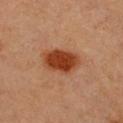<tbp_lesion>
<biopsy_status>not biopsied; imaged during a skin examination</biopsy_status>
<site>chest</site>
<patient>
  <sex>female</sex>
  <age_approx>45</age_approx>
</patient>
<image>
  <source>total-body photography crop</source>
  <field_of_view_mm>15</field_of_view_mm>
</image>
<lesion_size>
  <long_diameter_mm_approx>4.5</long_diameter_mm_approx>
</lesion_size>
<lighting>cross-polarized</lighting>
</tbp_lesion>